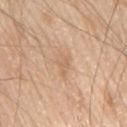Impression:
Captured during whole-body skin photography for melanoma surveillance; the lesion was not biopsied.
Context:
The patient is a male aged approximately 80. The lesion's longest dimension is about 3.5 mm. Automated image analysis of the tile measured a mean CIELAB color near L≈64 a*≈18 b*≈33. And it measured a border-irregularity index near 3.5/10 and a within-lesion color-variation index near 2/10. Cropped from a total-body skin-imaging series; the visible field is about 15 mm. On the mid back.